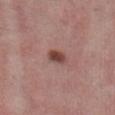{
  "biopsy_status": "not biopsied; imaged during a skin examination",
  "image": {
    "source": "total-body photography crop",
    "field_of_view_mm": 15
  },
  "patient": {
    "sex": "female",
    "age_approx": 55
  },
  "lesion_size": {
    "long_diameter_mm_approx": 2.5
  },
  "site": "abdomen",
  "lighting": "white-light"
}